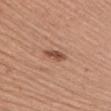workup: total-body-photography surveillance lesion; no biopsy
tile lighting: white-light
patient: male, approximately 55 years of age
body site: the arm
automated metrics: a border-irregularity index near 2/10 and radial color variation of about 0.5
image source: 15 mm crop, total-body photography
size: ~3 mm (longest diameter)